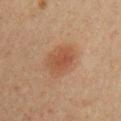No biopsy was performed on this lesion — it was imaged during a full skin examination and was not determined to be concerning.
Automated image analysis of the tile measured a border-irregularity index near 1.5/10, internal color variation of about 3 on a 0–10 scale, and radial color variation of about 1.
The recorded lesion diameter is about 4.5 mm.
Located on the chest.
The patient is a female approximately 40 years of age.
The tile uses cross-polarized illumination.
A 15 mm crop from a total-body photograph taken for skin-cancer surveillance.